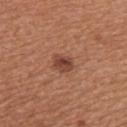Imaged during a routine full-body skin examination; the lesion was not biopsied and no histopathology is available.